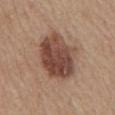The lesion was photographed on a routine skin check and not biopsied; there is no pathology result.
An algorithmic analysis of the crop reported an area of roughly 25 mm² and an eccentricity of roughly 0.65. It also reported an average lesion color of about L≈46 a*≈20 b*≈26 (CIELAB) and roughly 14 lightness units darker than nearby skin.
From the chest.
A male patient approximately 65 years of age.
This image is a 15 mm lesion crop taken from a total-body photograph.
The tile uses white-light illumination.